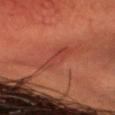Case summary:
- workup: total-body-photography surveillance lesion; no biopsy
- patient: male, approximately 50 years of age
- size: ~3 mm (longest diameter)
- lighting: cross-polarized illumination
- body site: the head or neck
- acquisition: ~15 mm tile from a whole-body skin photo